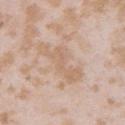lighting: white-light
site: left upper arm
image:
  source: total-body photography crop
  field_of_view_mm: 15
lesion_size:
  long_diameter_mm_approx: 6.0
patient:
  sex: female
  age_approx: 25